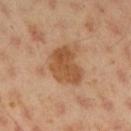The lesion was photographed on a routine skin check and not biopsied; there is no pathology result.
The lesion-visualizer software estimated a border-irregularity rating of about 3.5/10 and a color-variation rating of about 3/10. And it measured an automated nevus-likeness rating near 25 out of 100 and a detector confidence of about 100 out of 100 that the crop contains a lesion.
The recorded lesion diameter is about 4.5 mm.
The lesion is on the right upper arm.
A 15 mm close-up extracted from a 3D total-body photography capture.
A male subject, aged around 45.